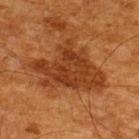No biopsy was performed on this lesion — it was imaged during a full skin examination and was not determined to be concerning.